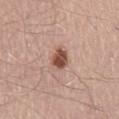Impression:
No biopsy was performed on this lesion — it was imaged during a full skin examination and was not determined to be concerning.
Clinical summary:
The lesion is on the mid back. This image is a 15 mm lesion crop taken from a total-body photograph. An algorithmic analysis of the crop reported a shape eccentricity near 0.5 and two-axis asymmetry of about 0.2. The software also gave a lesion–skin lightness drop of about 16 and a lesion-to-skin contrast of about 11 (normalized; higher = more distinct). And it measured a border-irregularity index near 2/10, internal color variation of about 3 on a 0–10 scale, and a peripheral color-asymmetry measure near 1. It also reported a nevus-likeness score of about 95/100 and lesion-presence confidence of about 100/100. This is a white-light tile. A male subject aged 58–62.